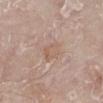Located on the right leg.
The patient is a male roughly 80 years of age.
A 15 mm crop from a total-body photograph taken for skin-cancer surveillance.
The tile uses white-light illumination.